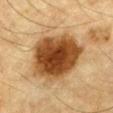{
  "biopsy_status": "not biopsied; imaged during a skin examination",
  "site": "chest",
  "lesion_size": {
    "long_diameter_mm_approx": 7.0
  },
  "patient": {
    "sex": "male",
    "age_approx": "85+"
  },
  "lighting": "cross-polarized",
  "image": {
    "source": "total-body photography crop",
    "field_of_view_mm": 15
  }
}